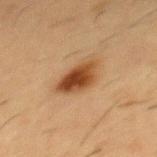The lesion was photographed on a routine skin check and not biopsied; there is no pathology result.